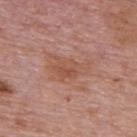From the back. A lesion tile, about 15 mm wide, cut from a 3D total-body photograph. A male patient in their mid- to late 70s.